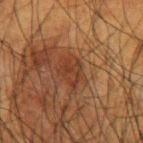Captured during whole-body skin photography for melanoma surveillance; the lesion was not biopsied. Automated tile analysis of the lesion measured a nevus-likeness score of about 0/100 and lesion-presence confidence of about 95/100. A close-up tile cropped from a whole-body skin photograph, about 15 mm across. A male patient aged around 60. Located on the arm. The tile uses cross-polarized illumination. The recorded lesion diameter is about 3.5 mm.